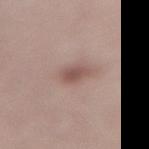biopsy_status: not biopsied; imaged during a skin examination
image:
  source: total-body photography crop
  field_of_view_mm: 15
lighting: white-light
lesion_size:
  long_diameter_mm_approx: 3.0
automated_metrics:
  area_mm2_approx: 4.5
  eccentricity: 0.8
  shape_asymmetry: 0.2
  cielab_L: 52
  cielab_a: 19
  cielab_b: 22
  vs_skin_darker_L: 10.0
  border_irregularity_0_10: 1.5
  nevus_likeness_0_100: 75
  lesion_detection_confidence_0_100: 100
patient:
  sex: female
  age_approx: 50
site: lower back